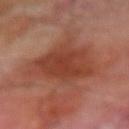Findings:
* patient — male, approximately 70 years of age
* image — total-body-photography crop, ~15 mm field of view
* location — the right forearm
* lesion size — about 8 mm
* TBP lesion metrics — a border-irregularity rating of about 4.5/10, a color-variation rating of about 3.5/10, and peripheral color asymmetry of about 1; an automated nevus-likeness rating near 5 out of 100 and a lesion-detection confidence of about 100/100
* illumination — cross-polarized